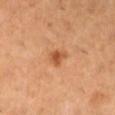Recorded during total-body skin imaging; not selected for excision or biopsy.
The subject is a female aged 28 to 32.
The tile uses cross-polarized illumination.
Measured at roughly 2.5 mm in maximum diameter.
From the left lower leg.
A close-up tile cropped from a whole-body skin photograph, about 15 mm across.
The total-body-photography lesion software estimated a lesion color around L≈55 a*≈27 b*≈40 in CIELAB, a lesion–skin lightness drop of about 11, and a normalized lesion–skin contrast near 7.5.